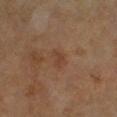Clinical impression: The lesion was photographed on a routine skin check and not biopsied; there is no pathology result. Background: From the left lower leg. The lesion-visualizer software estimated a footprint of about 3.5 mm² and two-axis asymmetry of about 0.3. It also reported a mean CIELAB color near L≈39 a*≈18 b*≈28, roughly 5 lightness units darker than nearby skin, and a lesion-to-skin contrast of about 5 (normalized; higher = more distinct). The analysis additionally found a border-irregularity index near 2.5/10 and a within-lesion color-variation index near 2/10. Imaged with cross-polarized lighting. A male subject aged around 65. A roughly 15 mm field-of-view crop from a total-body skin photograph.